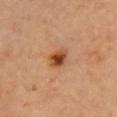Case summary:
– follow-up — total-body-photography surveillance lesion; no biopsy
– body site — the chest
– image — ~15 mm crop, total-body skin-cancer survey
– subject — male, in their mid- to late 60s